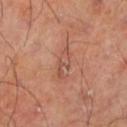biopsy_status: not biopsied; imaged during a skin examination
lighting: cross-polarized
site: left lower leg
patient:
  sex: male
  age_approx: 70
image:
  source: total-body photography crop
  field_of_view_mm: 15